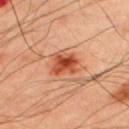Case summary:
- TBP lesion metrics — an area of roughly 7 mm², an eccentricity of roughly 0.5, and two-axis asymmetry of about 0.25; a lesion–skin lightness drop of about 14 and a normalized border contrast of about 10; a lesion-detection confidence of about 100/100
- patient — male, aged 48 to 52
- lighting — cross-polarized illumination
- acquisition — ~15 mm tile from a whole-body skin photo
- site — the upper back